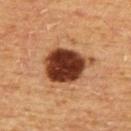Recorded during total-body skin imaging; not selected for excision or biopsy. The lesion's longest dimension is about 6 mm. Captured under cross-polarized illumination. A 15 mm close-up tile from a total-body photography series done for melanoma screening. A male patient about 65 years old. On the upper back.